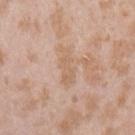biopsy status: no biopsy performed (imaged during a skin exam) | image-analysis metrics: a mean CIELAB color near L≈63 a*≈17 b*≈31, a lesion–skin lightness drop of about 6, and a lesion-to-skin contrast of about 5 (normalized; higher = more distinct); a border-irregularity rating of about 4.5/10 and peripheral color asymmetry of about 0.5 | tile lighting: white-light illumination | acquisition: ~15 mm crop, total-body skin-cancer survey | site: the left upper arm | patient: female, roughly 25 years of age.